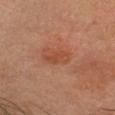Q: Was a biopsy performed?
A: total-body-photography surveillance lesion; no biopsy
Q: What are the patient's age and sex?
A: male, roughly 45 years of age
Q: How large is the lesion?
A: ≈3 mm
Q: Lesion location?
A: the head or neck
Q: Automated lesion metrics?
A: an area of roughly 4 mm², an outline eccentricity of about 0.85 (0 = round, 1 = elongated), and a shape-asymmetry score of about 0.35 (0 = symmetric); an average lesion color of about L≈40 a*≈23 b*≈29 (CIELAB) and a normalized lesion–skin contrast near 6; border irregularity of about 3.5 on a 0–10 scale and a peripheral color-asymmetry measure near 0.5
Q: What is the imaging modality?
A: ~15 mm crop, total-body skin-cancer survey
Q: Illumination type?
A: cross-polarized